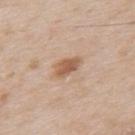biopsy status: catalogued during a skin exam; not biopsied | image-analysis metrics: a lesion area of about 5.5 mm² and a symmetry-axis asymmetry near 0.2; a lesion color around L≈58 a*≈19 b*≈32 in CIELAB, a lesion–skin lightness drop of about 11, and a lesion-to-skin contrast of about 8 (normalized; higher = more distinct); a classifier nevus-likeness of about 70/100 | anatomic site: the upper back | tile lighting: white-light | patient: male, aged around 65 | lesion diameter: ~3.5 mm (longest diameter) | acquisition: 15 mm crop, total-body photography.